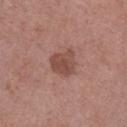<case>
  <biopsy_status>not biopsied; imaged during a skin examination</biopsy_status>
  <lighting>white-light</lighting>
  <patient>
    <sex>male</sex>
    <age_approx>55</age_approx>
  </patient>
  <image>
    <source>total-body photography crop</source>
    <field_of_view_mm>15</field_of_view_mm>
  </image>
  <site>right lower leg</site>
  <automated_metrics>
    <cielab_L>47</cielab_L>
    <cielab_a>23</cielab_a>
    <cielab_b>25</cielab_b>
    <vs_skin_darker_L>10.0</vs_skin_darker_L>
    <vs_skin_contrast_norm>7.5</vs_skin_contrast_norm>
    <border_irregularity_0_10>3.0</border_irregularity_0_10>
    <color_variation_0_10>2.5</color_variation_0_10>
    <peripheral_color_asymmetry>1.0</peripheral_color_asymmetry>
  </automated_metrics>
</case>